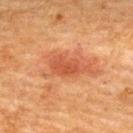Assessment:
Part of a total-body skin-imaging series; this lesion was reviewed on a skin check and was not flagged for biopsy.
Clinical summary:
Cropped from a whole-body photographic skin survey; the tile spans about 15 mm. A male subject, approximately 50 years of age. The total-body-photography lesion software estimated a lesion area of about 8 mm², a shape eccentricity near 0.85, and a symmetry-axis asymmetry near 0.25. The analysis additionally found a lesion color around L≈44 a*≈27 b*≈33 in CIELAB, roughly 9 lightness units darker than nearby skin, and a normalized border contrast of about 7. The software also gave an automated nevus-likeness rating near 100 out of 100. This is a cross-polarized tile. Located on the back.